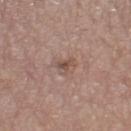Clinical impression: This lesion was catalogued during total-body skin photography and was not selected for biopsy. Acquisition and patient details: A roughly 15 mm field-of-view crop from a total-body skin photograph. The total-body-photography lesion software estimated a border-irregularity rating of about 4.5/10, a within-lesion color-variation index near 3/10, and peripheral color asymmetry of about 1. And it measured lesion-presence confidence of about 100/100. Imaged with white-light lighting. A female subject, roughly 65 years of age. The recorded lesion diameter is about 2.5 mm. On the left thigh.